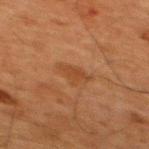Imaged during a routine full-body skin examination; the lesion was not biopsied and no histopathology is available.
A male subject, aged around 65.
The tile uses cross-polarized illumination.
The lesion's longest dimension is about 3.5 mm.
A 15 mm crop from a total-body photograph taken for skin-cancer surveillance.
On the upper back.